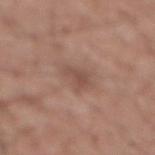biopsy status: imaged on a skin check; not biopsied | patient: male, aged around 75 | automated metrics: a lesion area of about 4.5 mm², a shape eccentricity near 0.8, and a symmetry-axis asymmetry near 0.4; an average lesion color of about L≈49 a*≈19 b*≈25 (CIELAB) and a lesion-to-skin contrast of about 6 (normalized; higher = more distinct); a border-irregularity rating of about 4.5/10, internal color variation of about 2.5 on a 0–10 scale, and peripheral color asymmetry of about 0.5 | acquisition: ~15 mm crop, total-body skin-cancer survey | location: the mid back | lesion size: ≈3 mm.